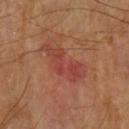Findings:
• notes — no biopsy performed (imaged during a skin exam)
• acquisition — 15 mm crop, total-body photography
• tile lighting — cross-polarized
• location — the arm
• lesion size — ≈6 mm